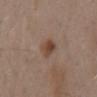Q: Is there a histopathology result?
A: imaged on a skin check; not biopsied
Q: Patient demographics?
A: male, aged 53–57
Q: Lesion size?
A: ≈2.5 mm
Q: Illumination type?
A: white-light
Q: What kind of image is this?
A: total-body-photography crop, ~15 mm field of view
Q: Lesion location?
A: the mid back